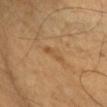Assessment: Part of a total-body skin-imaging series; this lesion was reviewed on a skin check and was not flagged for biopsy.